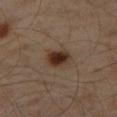{"biopsy_status": "not biopsied; imaged during a skin examination", "site": "right thigh", "automated_metrics": {"cielab_L": 28, "cielab_a": 14, "cielab_b": 23, "vs_skin_darker_L": 11.0, "vs_skin_contrast_norm": 11.5, "border_irregularity_0_10": 2.0, "color_variation_0_10": 4.0, "peripheral_color_asymmetry": 1.0, "nevus_likeness_0_100": 100, "lesion_detection_confidence_0_100": 100}, "patient": {"sex": "male", "age_approx": 60}, "image": {"source": "total-body photography crop", "field_of_view_mm": 15}, "lighting": "cross-polarized"}